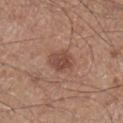  biopsy_status: not biopsied; imaged during a skin examination
  automated_metrics:
    cielab_L: 46
    cielab_a: 22
    cielab_b: 26
    vs_skin_contrast_norm: 7.5
    nevus_likeness_0_100: 80
    lesion_detection_confidence_0_100: 100
  patient:
    sex: male
    age_approx: 60
  lesion_size:
    long_diameter_mm_approx: 3.0
  lighting: white-light
  site: right lower leg
  image:
    source: total-body photography crop
    field_of_view_mm: 15